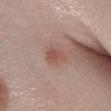This lesion was catalogued during total-body skin photography and was not selected for biopsy.
Cropped from a total-body skin-imaging series; the visible field is about 15 mm.
A female patient in their mid-60s.
The lesion is located on the right lower leg.
Captured under white-light illumination.